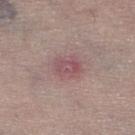| key | value |
|---|---|
| biopsy status | imaged on a skin check; not biopsied |
| anatomic site | the right lower leg |
| subject | female, aged around 65 |
| acquisition | 15 mm crop, total-body photography |